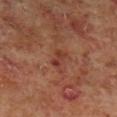Q: Was a biopsy performed?
A: imaged on a skin check; not biopsied
Q: Where on the body is the lesion?
A: the right lower leg
Q: What are the patient's age and sex?
A: male, aged 58 to 62
Q: What kind of image is this?
A: 15 mm crop, total-body photography